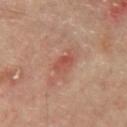Q: Was this lesion biopsied?
A: total-body-photography surveillance lesion; no biopsy
Q: What is the lesion's diameter?
A: ≈2.5 mm
Q: What is the anatomic site?
A: the mid back
Q: What are the patient's age and sex?
A: male, approximately 65 years of age
Q: How was this image acquired?
A: ~15 mm tile from a whole-body skin photo